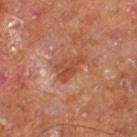workup = catalogued during a skin exam; not biopsied | patient = male, aged 63 to 67 | location = the left thigh | image source = ~15 mm crop, total-body skin-cancer survey.